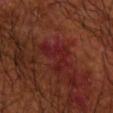Part of a total-body skin-imaging series; this lesion was reviewed on a skin check and was not flagged for biopsy. A 15 mm crop from a total-body photograph taken for skin-cancer surveillance. The lesion's longest dimension is about 4 mm. Imaged with cross-polarized lighting. An algorithmic analysis of the crop reported a footprint of about 9.5 mm², an eccentricity of roughly 0.55, and a shape-asymmetry score of about 0.7 (0 = symmetric). And it measured roughly 5 lightness units darker than nearby skin and a normalized lesion–skin contrast near 5.5. And it measured a nevus-likeness score of about 0/100 and lesion-presence confidence of about 65/100. From the right upper arm. A male patient, aged approximately 65.